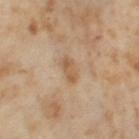The lesion was photographed on a routine skin check and not biopsied; there is no pathology result. An algorithmic analysis of the crop reported a border-irregularity index near 2.5/10 and radial color variation of about 0.5. The software also gave a lesion-detection confidence of about 100/100. The lesion is on the left thigh. A 15 mm close-up tile from a total-body photography series done for melanoma screening. The patient is a female about 55 years old. Captured under cross-polarized illumination.